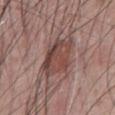biopsy status: imaged on a skin check; not biopsied.